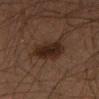Q: What is the lesion's diameter?
A: about 5 mm
Q: How was the tile lit?
A: cross-polarized
Q: How was this image acquired?
A: ~15 mm tile from a whole-body skin photo
Q: Who is the patient?
A: male, aged 63 to 67
Q: Automated lesion metrics?
A: a border-irregularity index near 3/10 and radial color variation of about 1
Q: Where on the body is the lesion?
A: the left thigh